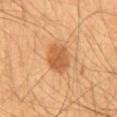Impression:
Recorded during total-body skin imaging; not selected for excision or biopsy.
Clinical summary:
From the back. Imaged with cross-polarized lighting. A lesion tile, about 15 mm wide, cut from a 3D total-body photograph. A male subject, aged 63–67. Measured at roughly 4 mm in maximum diameter.